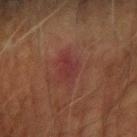Imaged during a routine full-body skin examination; the lesion was not biopsied and no histopathology is available. The tile uses cross-polarized illumination. The subject is a male roughly 70 years of age. Cropped from a whole-body photographic skin survey; the tile spans about 15 mm. The total-body-photography lesion software estimated a footprint of about 7 mm² and a shape-asymmetry score of about 0.25 (0 = symmetric). And it measured a lesion–skin lightness drop of about 5 and a normalized border contrast of about 6. The analysis additionally found an automated nevus-likeness rating near 0 out of 100. On the right forearm.